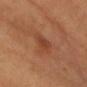Imaged during a routine full-body skin examination; the lesion was not biopsied and no histopathology is available.
Captured under cross-polarized illumination.
The lesion is located on the head or neck.
A female subject aged around 65.
Longest diameter approximately 2.5 mm.
A 15 mm crop from a total-body photograph taken for skin-cancer surveillance.